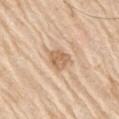Assessment: Recorded during total-body skin imaging; not selected for excision or biopsy. Background: Automated image analysis of the tile measured an average lesion color of about L≈64 a*≈18 b*≈34 (CIELAB), roughly 11 lightness units darker than nearby skin, and a normalized lesion–skin contrast near 7. The analysis additionally found internal color variation of about 2.5 on a 0–10 scale and radial color variation of about 1. The tile uses white-light illumination. A roughly 15 mm field-of-view crop from a total-body skin photograph. Located on the right upper arm. A male subject roughly 80 years of age.